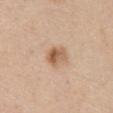biopsy status: total-body-photography surveillance lesion; no biopsy | anatomic site: the abdomen | lesion diameter: about 3 mm | subject: male, in their mid-60s | image source: total-body-photography crop, ~15 mm field of view | TBP lesion metrics: a lesion area of about 5.5 mm², an eccentricity of roughly 0.65, and a shape-asymmetry score of about 0.2 (0 = symmetric); border irregularity of about 2 on a 0–10 scale and peripheral color asymmetry of about 3.